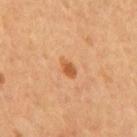- biopsy status — total-body-photography surveillance lesion; no biopsy
- imaging modality — ~15 mm tile from a whole-body skin photo
- automated metrics — a border-irregularity index near 2/10, a within-lesion color-variation index near 1.5/10, and a peripheral color-asymmetry measure near 0.5
- site — the back
- subject — male, aged around 55
- lesion size — about 2.5 mm
- illumination — cross-polarized